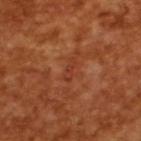Clinical impression: This lesion was catalogued during total-body skin photography and was not selected for biopsy. Background: A 15 mm close-up extracted from a 3D total-body photography capture. A male patient aged approximately 65.